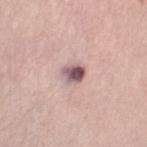diameter = about 2.5 mm | illumination = white-light | patient = female, aged 63 to 67 | imaging modality = total-body-photography crop, ~15 mm field of view | site = the right thigh.